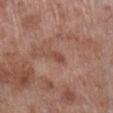lesion size: ≈2.5 mm
subject: male, aged around 70
body site: the leg
acquisition: ~15 mm tile from a whole-body skin photo
lighting: white-light illumination
TBP lesion metrics: a lesion color around L≈48 a*≈24 b*≈27 in CIELAB and about 8 CIELAB-L* units darker than the surrounding skin; a border-irregularity index near 3.5/10, a color-variation rating of about 0.5/10, and peripheral color asymmetry of about 0; a nevus-likeness score of about 0/100 and lesion-presence confidence of about 100/100